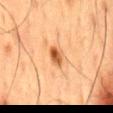{"biopsy_status": "not biopsied; imaged during a skin examination", "patient": {"sex": "male", "age_approx": 60}, "lighting": "cross-polarized", "site": "mid back", "image": {"source": "total-body photography crop", "field_of_view_mm": 15}}